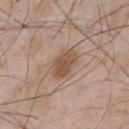Impression:
Captured during whole-body skin photography for melanoma surveillance; the lesion was not biopsied.
Image and clinical context:
A male patient aged around 50. The lesion is on the chest. Approximately 4 mm at its widest. Imaged with white-light lighting. A 15 mm crop from a total-body photograph taken for skin-cancer surveillance. Automated tile analysis of the lesion measured an average lesion color of about L≈52 a*≈18 b*≈29 (CIELAB), a lesion–skin lightness drop of about 10, and a lesion-to-skin contrast of about 8 (normalized; higher = more distinct). The analysis additionally found a nevus-likeness score of about 85/100.